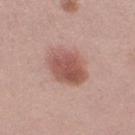Assessment: The lesion was photographed on a routine skin check and not biopsied; there is no pathology result. Image and clinical context: On the right lower leg. A female subject in their 20s. A roughly 15 mm field-of-view crop from a total-body skin photograph.